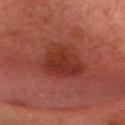No biopsy was performed on this lesion — it was imaged during a full skin examination and was not determined to be concerning.
A male patient roughly 55 years of age.
This is a cross-polarized tile.
Located on the head or neck.
The total-body-photography lesion software estimated a mean CIELAB color near L≈28 a*≈25 b*≈25 and roughly 7 lightness units darker than nearby skin.
Cropped from a total-body skin-imaging series; the visible field is about 15 mm.
Approximately 5.5 mm at its widest.